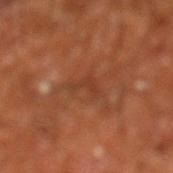The lesion is located on the left lower leg.
The tile uses cross-polarized illumination.
A 15 mm crop from a total-body photograph taken for skin-cancer surveillance.
Longest diameter approximately 2.5 mm.
The patient is a male in their mid-60s.